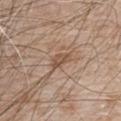Recorded during total-body skin imaging; not selected for excision or biopsy. The lesion-visualizer software estimated a mean CIELAB color near L≈51 a*≈18 b*≈29, roughly 9 lightness units darker than nearby skin, and a normalized border contrast of about 6.5. The analysis additionally found a border-irregularity rating of about 3/10 and radial color variation of about 0.5. The software also gave a nevus-likeness score of about 0/100 and a detector confidence of about 95 out of 100 that the crop contains a lesion. A region of skin cropped from a whole-body photographic capture, roughly 15 mm wide. A male subject approximately 80 years of age. The tile uses white-light illumination. On the chest.